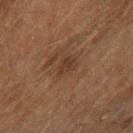A 15 mm crop from a total-body photograph taken for skin-cancer surveillance. Captured under cross-polarized illumination. About 2.5 mm across. From the right forearm. A male subject, about 75 years old.